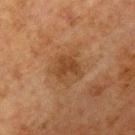Q: Was this lesion biopsied?
A: catalogued during a skin exam; not biopsied
Q: Where on the body is the lesion?
A: the chest
Q: Patient demographics?
A: male, aged around 65
Q: How was this image acquired?
A: ~15 mm tile from a whole-body skin photo
Q: What lighting was used for the tile?
A: cross-polarized illumination
Q: Lesion size?
A: ~3 mm (longest diameter)
Q: What did automated image analysis measure?
A: a lesion area of about 5 mm² and two-axis asymmetry of about 0.45; a mean CIELAB color near L≈37 a*≈19 b*≈31 and about 7 CIELAB-L* units darker than the surrounding skin; internal color variation of about 2 on a 0–10 scale and a peripheral color-asymmetry measure near 0.5